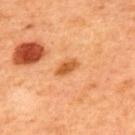Findings:
- imaging modality · ~15 mm crop, total-body skin-cancer survey
- anatomic site · the upper back
- subject · male, aged 48 to 52
- lighting · cross-polarized
- lesion size · ~3 mm (longest diameter)